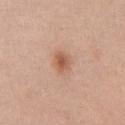The lesion was tiled from a total-body skin photograph and was not biopsied. Imaged with white-light lighting. Automated image analysis of the tile measured a lesion area of about 5 mm², an eccentricity of roughly 0.7, and a shape-asymmetry score of about 0.2 (0 = symmetric). It also reported an average lesion color of about L≈58 a*≈22 b*≈32 (CIELAB), a lesion–skin lightness drop of about 10, and a normalized lesion–skin contrast near 7.5. And it measured border irregularity of about 2 on a 0–10 scale, internal color variation of about 3.5 on a 0–10 scale, and radial color variation of about 1. It also reported a nevus-likeness score of about 90/100 and lesion-presence confidence of about 100/100. A 15 mm crop from a total-body photograph taken for skin-cancer surveillance. From the chest. A female patient aged 38 to 42. About 3 mm across.